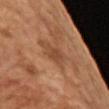Imaged during a routine full-body skin examination; the lesion was not biopsied and no histopathology is available.
A close-up tile cropped from a whole-body skin photograph, about 15 mm across.
A male subject roughly 65 years of age.